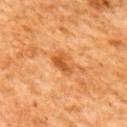Assessment:
Captured during whole-body skin photography for melanoma surveillance; the lesion was not biopsied.
Context:
The lesion is located on the back. This is a cross-polarized tile. The total-body-photography lesion software estimated a footprint of about 6.5 mm², an eccentricity of roughly 0.8, and a symmetry-axis asymmetry near 0.15. The software also gave a lesion–skin lightness drop of about 11 and a normalized lesion–skin contrast near 7.5. And it measured a classifier nevus-likeness of about 20/100. Approximately 3.5 mm at its widest. This image is a 15 mm lesion crop taken from a total-body photograph. A female subject, in their mid- to late 60s.